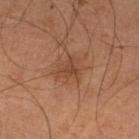Impression:
Part of a total-body skin-imaging series; this lesion was reviewed on a skin check and was not flagged for biopsy.